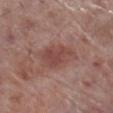Q: Is there a histopathology result?
A: catalogued during a skin exam; not biopsied
Q: Who is the patient?
A: male, in their 70s
Q: What kind of image is this?
A: 15 mm crop, total-body photography
Q: What did automated image analysis measure?
A: roughly 8 lightness units darker than nearby skin and a normalized border contrast of about 6.5; border irregularity of about 3 on a 0–10 scale, internal color variation of about 2.5 on a 0–10 scale, and radial color variation of about 1
Q: What is the anatomic site?
A: the right lower leg
Q: Lesion size?
A: about 5 mm